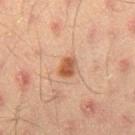biopsy_status: not biopsied; imaged during a skin examination
automated_metrics:
  area_mm2_approx: 4.0
  shape_asymmetry: 0.2
  vs_skin_darker_L: 11.0
  border_irregularity_0_10: 1.5
  color_variation_0_10: 3.0
  peripheral_color_asymmetry: 1.0
  nevus_likeness_0_100: 95
  lesion_detection_confidence_0_100: 100
patient:
  sex: male
  age_approx: 45
image:
  source: total-body photography crop
  field_of_view_mm: 15
lesion_size:
  long_diameter_mm_approx: 2.5
site: leg
lighting: cross-polarized